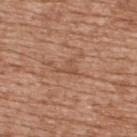biopsy_status: not biopsied; imaged during a skin examination
patient:
  sex: male
  age_approx: 60
lesion_size:
  long_diameter_mm_approx: 2.5
lighting: white-light
site: upper back
automated_metrics:
  cielab_L: 51
  cielab_a: 22
  cielab_b: 32
  vs_skin_darker_L: 7.0
  nevus_likeness_0_100: 0
  lesion_detection_confidence_0_100: 55
image:
  source: total-body photography crop
  field_of_view_mm: 15